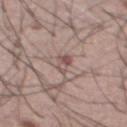No biopsy was performed on this lesion — it was imaged during a full skin examination and was not determined to be concerning. A male subject, about 55 years old. A region of skin cropped from a whole-body photographic capture, roughly 15 mm wide. Located on the chest.